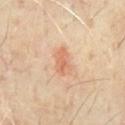Clinical impression:
This lesion was catalogued during total-body skin photography and was not selected for biopsy.
Clinical summary:
A male subject aged 63–67. Located on the chest. This image is a 15 mm lesion crop taken from a total-body photograph. This is a cross-polarized tile.